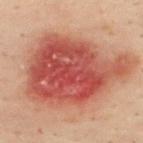Clinical impression:
Recorded during total-body skin imaging; not selected for excision or biopsy.
Image and clinical context:
A male subject about 50 years old. This is a cross-polarized tile. The lesion is located on the upper back. The lesion's longest dimension is about 12 mm. A 15 mm close-up tile from a total-body photography series done for melanoma screening.